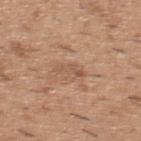Notes:
- anatomic site · the upper back
- patient · male, approximately 40 years of age
- diameter · about 3 mm
- image · total-body-photography crop, ~15 mm field of view